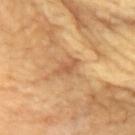workup = no biopsy performed (imaged during a skin exam)
tile lighting = cross-polarized illumination
anatomic site = the chest
size = about 3 mm
TBP lesion metrics = a nevus-likeness score of about 0/100
image = ~15 mm crop, total-body skin-cancer survey
subject = female